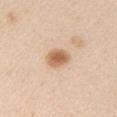follow-up=no biopsy performed (imaged during a skin exam)
acquisition=15 mm crop, total-body photography
lesion size=≈3 mm
patient=male, aged 58 to 62
location=the right upper arm
illumination=white-light illumination